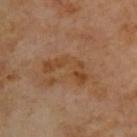Impression: Recorded during total-body skin imaging; not selected for excision or biopsy. Background: A male subject about 70 years old. Captured under cross-polarized illumination. Measured at roughly 6 mm in maximum diameter. A roughly 15 mm field-of-view crop from a total-body skin photograph. The lesion is located on the upper back.